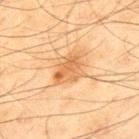biopsy status = catalogued during a skin exam; not biopsied | automated lesion analysis = border irregularity of about 2.5 on a 0–10 scale and internal color variation of about 4 on a 0–10 scale | tile lighting = cross-polarized | size = ≈4 mm | subject = male, in their mid-40s | acquisition = 15 mm crop, total-body photography | body site = the mid back.